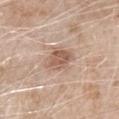Captured under white-light illumination.
On the front of the torso.
A male patient roughly 80 years of age.
A 15 mm crop from a total-body photograph taken for skin-cancer surveillance.